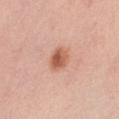Impression: Imaged during a routine full-body skin examination; the lesion was not biopsied and no histopathology is available. Image and clinical context: Approximately 3 mm at its widest. The subject is a female about 35 years old. An algorithmic analysis of the crop reported a border-irregularity index near 1.5/10 and peripheral color asymmetry of about 1.5. And it measured a classifier nevus-likeness of about 100/100. Located on the left thigh. Captured under white-light illumination. This image is a 15 mm lesion crop taken from a total-body photograph.